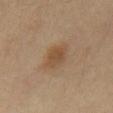Imaged during a routine full-body skin examination; the lesion was not biopsied and no histopathology is available.
The lesion's longest dimension is about 4 mm.
A male patient about 60 years old.
The lesion-visualizer software estimated a border-irregularity index near 3/10 and radial color variation of about 1. The software also gave a detector confidence of about 100 out of 100 that the crop contains a lesion.
A lesion tile, about 15 mm wide, cut from a 3D total-body photograph.
On the mid back.